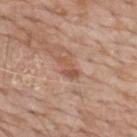Part of a total-body skin-imaging series; this lesion was reviewed on a skin check and was not flagged for biopsy.
About 3 mm across.
Located on the mid back.
A male patient, in their 60s.
A roughly 15 mm field-of-view crop from a total-body skin photograph.
The tile uses white-light illumination.